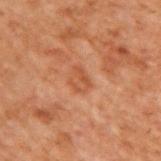Assessment: Imaged during a routine full-body skin examination; the lesion was not biopsied and no histopathology is available. Clinical summary: An algorithmic analysis of the crop reported a lesion area of about 4.5 mm², an eccentricity of roughly 0.65, and two-axis asymmetry of about 0.25. The software also gave a mean CIELAB color near L≈40 a*≈21 b*≈30, a lesion–skin lightness drop of about 6, and a normalized lesion–skin contrast near 5.5. And it measured border irregularity of about 2.5 on a 0–10 scale and a color-variation rating of about 3/10. The software also gave a classifier nevus-likeness of about 0/100 and a detector confidence of about 100 out of 100 that the crop contains a lesion. Captured under cross-polarized illumination. A region of skin cropped from a whole-body photographic capture, roughly 15 mm wide. A male subject, approximately 70 years of age. From the upper back.